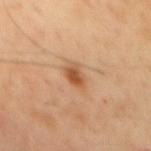Recorded during total-body skin imaging; not selected for excision or biopsy.
Cropped from a whole-body photographic skin survey; the tile spans about 15 mm.
Imaged with cross-polarized lighting.
A male patient aged 43–47.
Located on the back.